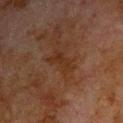The lesion was tiled from a total-body skin photograph and was not biopsied. The recorded lesion diameter is about 3.5 mm. A male patient aged 78 to 82. The tile uses cross-polarized illumination. Cropped from a total-body skin-imaging series; the visible field is about 15 mm. From the chest.